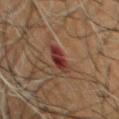anatomic site=the front of the torso
lesion size=≈3 mm
subject=male, aged around 65
automated metrics=a lesion area of about 5.5 mm², an eccentricity of roughly 0.7, and a symmetry-axis asymmetry near 0.2; a nevus-likeness score of about 0/100 and lesion-presence confidence of about 100/100
image source=15 mm crop, total-body photography